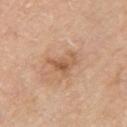Clinical impression: The lesion was tiled from a total-body skin photograph and was not biopsied. Acquisition and patient details: Imaged with white-light lighting. From the right upper arm. The lesion's longest dimension is about 4 mm. Automated image analysis of the tile measured a lesion color around L≈58 a*≈20 b*≈34 in CIELAB, a lesion–skin lightness drop of about 9, and a normalized lesion–skin contrast near 6.5. And it measured a border-irregularity rating of about 6/10, a within-lesion color-variation index near 4.5/10, and radial color variation of about 1.5. A male subject aged approximately 80. Cropped from a whole-body photographic skin survey; the tile spans about 15 mm.